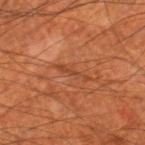| feature | finding |
|---|---|
| notes | imaged on a skin check; not biopsied |
| acquisition | total-body-photography crop, ~15 mm field of view |
| body site | the right thigh |
| patient | male, in their 70s |